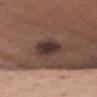This lesion was catalogued during total-body skin photography and was not selected for biopsy. This is a white-light tile. A 15 mm close-up extracted from a 3D total-body photography capture. The patient is a female approximately 50 years of age. On the chest. Automated image analysis of the tile measured an average lesion color of about L≈34 a*≈15 b*≈19 (CIELAB) and a normalized lesion–skin contrast near 10.5. It also reported a detector confidence of about 100 out of 100 that the crop contains a lesion.